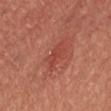Measured at roughly 2.5 mm in maximum diameter. A male subject aged around 45. On the head or neck. A close-up tile cropped from a whole-body skin photograph, about 15 mm across.